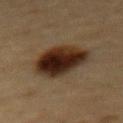biopsy status: catalogued during a skin exam; not biopsied | subject: male, approximately 85 years of age or older | lesion diameter: ≈5.5 mm | lighting: cross-polarized illumination | site: the abdomen | acquisition: total-body-photography crop, ~15 mm field of view.